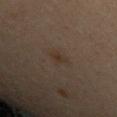• follow-up · imaged on a skin check; not biopsied
• site · the left upper arm
• image source · ~15 mm tile from a whole-body skin photo
• automated lesion analysis · a footprint of about 3.5 mm², a shape eccentricity near 0.75, and a shape-asymmetry score of about 0.3 (0 = symmetric); a mean CIELAB color near L≈25 a*≈10 b*≈19, about 4 CIELAB-L* units darker than the surrounding skin, and a lesion-to-skin contrast of about 5.5 (normalized; higher = more distinct); a lesion-detection confidence of about 100/100
• diameter · ~2.5 mm (longest diameter)
• subject · female, approximately 30 years of age
• lighting · cross-polarized